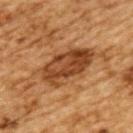Case summary:
• workup — catalogued during a skin exam; not biopsied
• acquisition — ~15 mm crop, total-body skin-cancer survey
• patient — male, aged 83 or over
• anatomic site — the upper back
• TBP lesion metrics — a lesion color around L≈38 a*≈21 b*≈33 in CIELAB and a lesion–skin lightness drop of about 11; a border-irregularity rating of about 3/10 and peripheral color asymmetry of about 2; an automated nevus-likeness rating near 45 out of 100 and a detector confidence of about 95 out of 100 that the crop contains a lesion
• size — ≈7 mm
• illumination — cross-polarized illumination